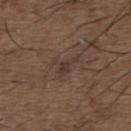notes — catalogued during a skin exam; not biopsied | site — the back | subject — male, aged approximately 50 | acquisition — ~15 mm tile from a whole-body skin photo.